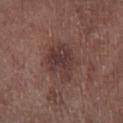No biopsy was performed on this lesion — it was imaged during a full skin examination and was not determined to be concerning. On the right lower leg. A 15 mm crop from a total-body photograph taken for skin-cancer surveillance. Automated tile analysis of the lesion measured an outline eccentricity of about 0.6 (0 = round, 1 = elongated) and two-axis asymmetry of about 0.25. It also reported an average lesion color of about L≈36 a*≈18 b*≈19 (CIELAB), a lesion–skin lightness drop of about 8, and a normalized lesion–skin contrast near 7.5. The analysis additionally found a border-irregularity rating of about 3/10, internal color variation of about 3.5 on a 0–10 scale, and a peripheral color-asymmetry measure near 1. Captured under white-light illumination. A male subject approximately 70 years of age.